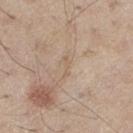- follow-up: catalogued during a skin exam; not biopsied
- body site: the right thigh
- subject: male, aged around 60
- image: total-body-photography crop, ~15 mm field of view